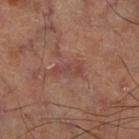Captured during whole-body skin photography for melanoma surveillance; the lesion was not biopsied.